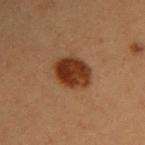Assessment:
Recorded during total-body skin imaging; not selected for excision or biopsy.
Acquisition and patient details:
The recorded lesion diameter is about 4.5 mm. The lesion is on the right upper arm. Imaged with cross-polarized lighting. A region of skin cropped from a whole-body photographic capture, roughly 15 mm wide. A female patient in their 40s.